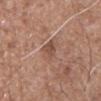Impression:
Imaged during a routine full-body skin examination; the lesion was not biopsied and no histopathology is available.
Background:
The patient is a male aged around 75. Imaged with white-light lighting. The lesion is on the mid back. A 15 mm close-up tile from a total-body photography series done for melanoma screening. The lesion-visualizer software estimated an average lesion color of about L≈52 a*≈19 b*≈27 (CIELAB), roughly 7 lightness units darker than nearby skin, and a lesion-to-skin contrast of about 5.5 (normalized; higher = more distinct). It also reported a border-irregularity rating of about 3/10 and a within-lesion color-variation index near 4.5/10.